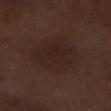Context:
The tile uses white-light illumination. The lesion is located on the right lower leg. A male subject, approximately 70 years of age. A region of skin cropped from a whole-body photographic capture, roughly 15 mm wide. Approximately 6 mm at its widest. The total-body-photography lesion software estimated a footprint of about 27 mm², a shape eccentricity near 0.35, and a symmetry-axis asymmetry near 0.15. The software also gave a border-irregularity rating of about 2/10, a within-lesion color-variation index near 3/10, and peripheral color asymmetry of about 1. It also reported a nevus-likeness score of about 10/100 and lesion-presence confidence of about 100/100.